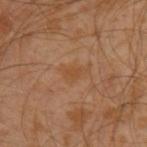| key | value |
|---|---|
| follow-up | imaged on a skin check; not biopsied |
| image | 15 mm crop, total-body photography |
| lesion size | ≈2.5 mm |
| location | the arm |
| patient | male, roughly 30 years of age |